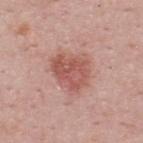Captured during whole-body skin photography for melanoma surveillance; the lesion was not biopsied.
Captured under white-light illumination.
An algorithmic analysis of the crop reported a lesion area of about 12 mm², a shape eccentricity near 0.7, and two-axis asymmetry of about 0.3. The analysis additionally found a mean CIELAB color near L≈54 a*≈26 b*≈26, roughly 10 lightness units darker than nearby skin, and a lesion-to-skin contrast of about 7 (normalized; higher = more distinct). The software also gave a within-lesion color-variation index near 4/10.
A 15 mm close-up extracted from a 3D total-body photography capture.
From the upper back.
The subject is a male aged approximately 50.
Longest diameter approximately 4.5 mm.